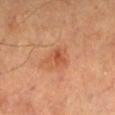Clinical impression: Captured during whole-body skin photography for melanoma surveillance; the lesion was not biopsied. Background: The lesion is located on the right lower leg. Approximately 3 mm at its widest. Captured under cross-polarized illumination. A male subject, aged 63–67. This image is a 15 mm lesion crop taken from a total-body photograph.